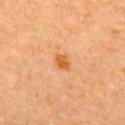No biopsy was performed on this lesion — it was imaged during a full skin examination and was not determined to be concerning. The lesion is on the back. This is a cross-polarized tile. A female subject about 30 years old. A close-up tile cropped from a whole-body skin photograph, about 15 mm across.